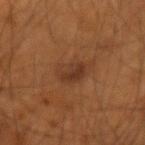Q: Who is the patient?
A: male, aged 53 to 57
Q: What lighting was used for the tile?
A: cross-polarized illumination
Q: How was this image acquired?
A: total-body-photography crop, ~15 mm field of view
Q: Automated lesion metrics?
A: internal color variation of about 4 on a 0–10 scale and a peripheral color-asymmetry measure near 1.5
Q: Lesion location?
A: the right forearm